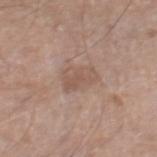Q: Is there a histopathology result?
A: imaged on a skin check; not biopsied
Q: Lesion location?
A: the right thigh
Q: Patient demographics?
A: male, aged 63 to 67
Q: How was this image acquired?
A: ~15 mm crop, total-body skin-cancer survey